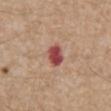Q: Was a biopsy performed?
A: imaged on a skin check; not biopsied
Q: What is the lesion's diameter?
A: ≈3 mm
Q: What are the patient's age and sex?
A: male, aged around 70
Q: What is the anatomic site?
A: the abdomen
Q: How was the tile lit?
A: white-light illumination
Q: How was this image acquired?
A: ~15 mm crop, total-body skin-cancer survey